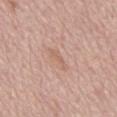Q: Who is the patient?
A: female, in their mid-60s
Q: What is the anatomic site?
A: the back
Q: Illumination type?
A: white-light illumination
Q: How large is the lesion?
A: ~3.5 mm (longest diameter)
Q: What is the imaging modality?
A: 15 mm crop, total-body photography